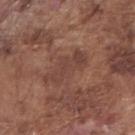follow-up: imaged on a skin check; not biopsied
image: 15 mm crop, total-body photography
location: the right forearm
illumination: white-light illumination
subject: male, in their mid-70s
size: ~5.5 mm (longest diameter)